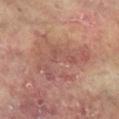Imaged during a routine full-body skin examination; the lesion was not biopsied and no histopathology is available.
The lesion is located on the right arm.
A roughly 15 mm field-of-view crop from a total-body skin photograph.
A female subject in their 80s.